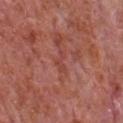Q: Was a biopsy performed?
A: no biopsy performed (imaged during a skin exam)
Q: Where on the body is the lesion?
A: the chest
Q: What is the imaging modality?
A: 15 mm crop, total-body photography
Q: What is the lesion's diameter?
A: ≈2.5 mm
Q: Patient demographics?
A: male, aged approximately 65